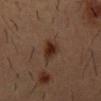Clinical impression: The lesion was photographed on a routine skin check and not biopsied; there is no pathology result. Clinical summary: The patient is a male aged approximately 55. The total-body-photography lesion software estimated a normalized border contrast of about 10.5. It also reported a border-irregularity index near 3/10, a within-lesion color-variation index near 3/10, and radial color variation of about 1. On the chest. The recorded lesion diameter is about 3 mm. A 15 mm close-up extracted from a 3D total-body photography capture.